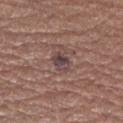| feature | finding |
|---|---|
| workup | catalogued during a skin exam; not biopsied |
| anatomic site | the right forearm |
| image source | ~15 mm crop, total-body skin-cancer survey |
| lesion diameter | about 2.5 mm |
| patient | female, aged 63–67 |
| illumination | white-light illumination |